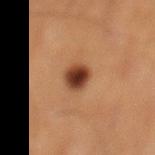notes: imaged on a skin check; not biopsied | TBP lesion metrics: a footprint of about 6 mm², an outline eccentricity of about 0.75 (0 = round, 1 = elongated), and a symmetry-axis asymmetry near 0.25; a classifier nevus-likeness of about 100/100 | image source: ~15 mm crop, total-body skin-cancer survey | location: the left lower leg | patient: male, aged around 60.